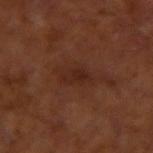The lesion was photographed on a routine skin check and not biopsied; there is no pathology result.
Located on the arm.
This image is a 15 mm lesion crop taken from a total-body photograph.
Captured under cross-polarized illumination.
A male patient, in their mid- to late 60s.
The recorded lesion diameter is about 3.5 mm.
The lesion-visualizer software estimated a mean CIELAB color near L≈23 a*≈20 b*≈24, roughly 5 lightness units darker than nearby skin, and a normalized border contrast of about 6.5. The software also gave a nevus-likeness score of about 0/100 and lesion-presence confidence of about 100/100.